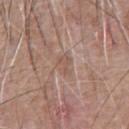Clinical impression:
Captured during whole-body skin photography for melanoma surveillance; the lesion was not biopsied.
Acquisition and patient details:
A male subject, approximately 60 years of age. Located on the chest. Imaged with white-light lighting. A roughly 15 mm field-of-view crop from a total-body skin photograph. An algorithmic analysis of the crop reported an area of roughly 4 mm², a shape eccentricity near 0.8, and a symmetry-axis asymmetry near 0.35. The software also gave an average lesion color of about L≈54 a*≈17 b*≈26 (CIELAB) and a normalized lesion–skin contrast near 4.5.